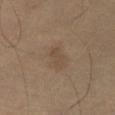Case summary:
* notes: total-body-photography surveillance lesion; no biopsy
* location: the abdomen
* imaging modality: total-body-photography crop, ~15 mm field of view
* TBP lesion metrics: a mean CIELAB color near L≈43 a*≈13 b*≈26, about 5 CIELAB-L* units darker than the surrounding skin, and a normalized lesion–skin contrast near 5; a nevus-likeness score of about 0/100 and a lesion-detection confidence of about 100/100
* tile lighting: cross-polarized
* patient: male, about 65 years old